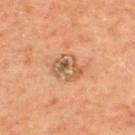Impression:
The lesion was photographed on a routine skin check and not biopsied; there is no pathology result.
Background:
The lesion is on the upper back. A 15 mm close-up tile from a total-body photography series done for melanoma screening. Imaged with cross-polarized lighting. The subject is a male aged 63–67.